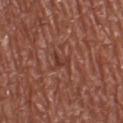Case summary:
* notes — imaged on a skin check; not biopsied
* imaging modality — ~15 mm tile from a whole-body skin photo
* patient — male, aged approximately 75
* lighting — white-light illumination
* anatomic site — the left lower leg